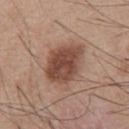  patient:
    sex: male
    age_approx: 55
  site: chest
  image:
    source: total-body photography crop
    field_of_view_mm: 15